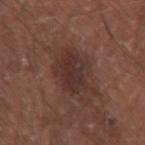Clinical impression: Part of a total-body skin-imaging series; this lesion was reviewed on a skin check and was not flagged for biopsy. Clinical summary: About 6.5 mm across. The patient is a male approximately 65 years of age. The lesion is on the right upper arm. Automated image analysis of the tile measured a lesion area of about 15 mm², an outline eccentricity of about 0.75 (0 = round, 1 = elongated), and two-axis asymmetry of about 0.25. It also reported a mean CIELAB color near L≈32 a*≈18 b*≈20, a lesion–skin lightness drop of about 8, and a lesion-to-skin contrast of about 8 (normalized; higher = more distinct). The software also gave a border-irregularity rating of about 3.5/10 and a within-lesion color-variation index near 4/10. The software also gave lesion-presence confidence of about 100/100. This is a white-light tile. This image is a 15 mm lesion crop taken from a total-body photograph.